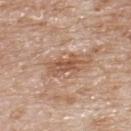* follow-up · total-body-photography surveillance lesion; no biopsy
* automated metrics · a color-variation rating of about 4/10 and a peripheral color-asymmetry measure near 1.5; a lesion-detection confidence of about 100/100
* imaging modality · ~15 mm crop, total-body skin-cancer survey
* body site · the upper back
* lesion size · about 4 mm
* patient · male, aged 78 to 82
* illumination · white-light